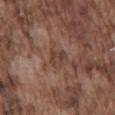Q: Is there a histopathology result?
A: catalogued during a skin exam; not biopsied